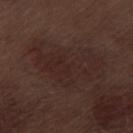<tbp_lesion>
  <lesion_size>
    <long_diameter_mm_approx>8.0</long_diameter_mm_approx>
  </lesion_size>
  <image>
    <source>total-body photography crop</source>
    <field_of_view_mm>15</field_of_view_mm>
  </image>
  <patient>
    <sex>male</sex>
    <age_approx>70</age_approx>
  </patient>
  <automated_metrics>
    <vs_skin_darker_L>4.0</vs_skin_darker_L>
    <vs_skin_contrast_norm>5.5</vs_skin_contrast_norm>
    <lesion_detection_confidence_0_100>95</lesion_detection_confidence_0_100>
  </automated_metrics>
  <lighting>white-light</lighting>
  <site>left thigh</site>
</tbp_lesion>